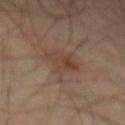follow-up: catalogued during a skin exam; not biopsied | anatomic site: the abdomen | image-analysis metrics: a mean CIELAB color near L≈41 a*≈16 b*≈24, about 6 CIELAB-L* units darker than the surrounding skin, and a normalized border contrast of about 5.5 | acquisition: total-body-photography crop, ~15 mm field of view | subject: male, aged 63–67 | lesion size: about 4 mm.